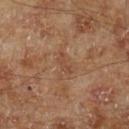The lesion was tiled from a total-body skin photograph and was not biopsied. On the leg. A male patient roughly 70 years of age. The lesion's longest dimension is about 3 mm. A 15 mm crop from a total-body photograph taken for skin-cancer surveillance.